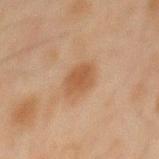Q: Was a biopsy performed?
A: imaged on a skin check; not biopsied
Q: Patient demographics?
A: male, aged 43 to 47
Q: How was this image acquired?
A: total-body-photography crop, ~15 mm field of view
Q: Illumination type?
A: cross-polarized illumination
Q: What is the anatomic site?
A: the back